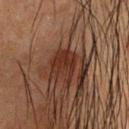Case summary:
* workup: catalogued during a skin exam; not biopsied
* imaging modality: ~15 mm crop, total-body skin-cancer survey
* lesion size: ≈4.5 mm
* location: the head or neck
* lighting: cross-polarized illumination
* subject: male, about 50 years old